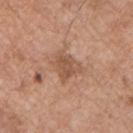lighting: white-light illumination | image source: 15 mm crop, total-body photography | TBP lesion metrics: an area of roughly 7.5 mm² and an outline eccentricity of about 0.55 (0 = round, 1 = elongated); an average lesion color of about L≈53 a*≈21 b*≈31 (CIELAB) and a normalized lesion–skin contrast near 6.5 | size: about 3.5 mm | body site: the chest | patient: male, in their mid-50s.